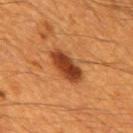Assessment: This lesion was catalogued during total-body skin photography and was not selected for biopsy. Background: Approximately 5 mm at its widest. A roughly 15 mm field-of-view crop from a total-body skin photograph. On the mid back. The tile uses cross-polarized illumination. A male patient aged around 60.